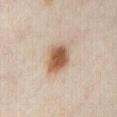Q: Was this lesion biopsied?
A: imaged on a skin check; not biopsied
Q: Lesion location?
A: the abdomen
Q: Who is the patient?
A: female, roughly 35 years of age
Q: How was the tile lit?
A: cross-polarized
Q: What is the imaging modality?
A: ~15 mm crop, total-body skin-cancer survey